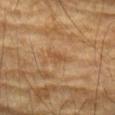Recorded during total-body skin imaging; not selected for excision or biopsy.
A lesion tile, about 15 mm wide, cut from a 3D total-body photograph.
From the left forearm.
Approximately 2.5 mm at its widest.
The tile uses cross-polarized illumination.
Automated tile analysis of the lesion measured a footprint of about 3 mm², an eccentricity of roughly 0.9, and two-axis asymmetry of about 0.3. And it measured border irregularity of about 3.5 on a 0–10 scale and internal color variation of about 1 on a 0–10 scale.
The subject is a male aged 83 or older.